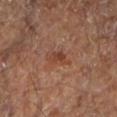Clinical impression: Imaged during a routine full-body skin examination; the lesion was not biopsied and no histopathology is available. Clinical summary: Automated image analysis of the tile measured an area of roughly 5 mm², a shape eccentricity near 0.85, and a symmetry-axis asymmetry near 0.4. The software also gave an average lesion color of about L≈42 a*≈22 b*≈29 (CIELAB), a lesion–skin lightness drop of about 7, and a normalized lesion–skin contrast near 6. The software also gave a within-lesion color-variation index near 3.5/10. A roughly 15 mm field-of-view crop from a total-body skin photograph. The lesion's longest dimension is about 3.5 mm. The patient is aged 63–67. On the left lower leg. Captured under cross-polarized illumination.